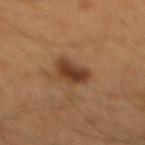<case>
  <site>back</site>
  <image>
    <source>total-body photography crop</source>
    <field_of_view_mm>15</field_of_view_mm>
  </image>
  <lighting>cross-polarized</lighting>
  <patient>
    <sex>male</sex>
    <age_approx>65</age_approx>
  </patient>
  <lesion_size>
    <long_diameter_mm_approx>3.5</long_diameter_mm_approx>
  </lesion_size>
</case>